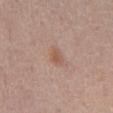Case summary:
- notes · imaged on a skin check; not biopsied
- subject · male, about 55 years old
- acquisition · ~15 mm crop, total-body skin-cancer survey
- anatomic site · the abdomen